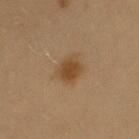notes=imaged on a skin check; not biopsied
anatomic site=the right upper arm
subject=female, aged around 25
illumination=cross-polarized illumination
image source=~15 mm tile from a whole-body skin photo
lesion size=≈3.5 mm
TBP lesion metrics=a footprint of about 6.5 mm², a shape eccentricity near 0.65, and two-axis asymmetry of about 0.2